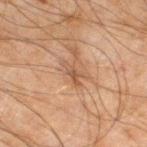A male subject aged around 45. The lesion is located on the left lower leg. A roughly 15 mm field-of-view crop from a total-body skin photograph. This is a cross-polarized tile. Automated image analysis of the tile measured a classifier nevus-likeness of about 0/100 and a lesion-detection confidence of about 90/100.